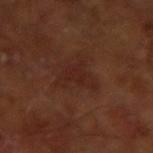On the right upper arm. About 4.5 mm across. A male subject, aged approximately 65. This is a cross-polarized tile. The total-body-photography lesion software estimated border irregularity of about 6 on a 0–10 scale, a color-variation rating of about 2/10, and radial color variation of about 0.5. The analysis additionally found an automated nevus-likeness rating near 0 out of 100. This image is a 15 mm lesion crop taken from a total-body photograph.